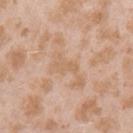Assessment:
Recorded during total-body skin imaging; not selected for excision or biopsy.
Clinical summary:
A 15 mm close-up tile from a total-body photography series done for melanoma screening. Approximately 3 mm at its widest. The subject is a female aged 23–27. The total-body-photography lesion software estimated a lesion color around L≈63 a*≈18 b*≈34 in CIELAB, a lesion–skin lightness drop of about 5, and a lesion-to-skin contrast of about 4.5 (normalized; higher = more distinct). The analysis additionally found a nevus-likeness score of about 0/100 and a detector confidence of about 100 out of 100 that the crop contains a lesion. The lesion is on the left upper arm.